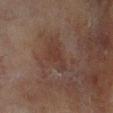No biopsy was performed on this lesion — it was imaged during a full skin examination and was not determined to be concerning.
On the left lower leg.
A female patient in their 80s.
A 15 mm close-up tile from a total-body photography series done for melanoma screening.
The recorded lesion diameter is about 4 mm.
Imaged with cross-polarized lighting.